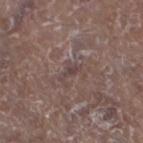* notes: no biopsy performed (imaged during a skin exam)
* patient: male, in their 80s
* body site: the left lower leg
* tile lighting: white-light
* image: ~15 mm crop, total-body skin-cancer survey